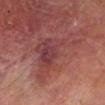This lesion was catalogued during total-body skin photography and was not selected for biopsy.
Located on the right lower leg.
This image is a 15 mm lesion crop taken from a total-body photograph.
A male patient, aged approximately 70.
About 8 mm across.